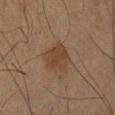The lesion was tiled from a total-body skin photograph and was not biopsied.
A 15 mm crop from a total-body photograph taken for skin-cancer surveillance.
The lesion is located on the left forearm.
Approximately 4 mm at its widest.
A male patient roughly 65 years of age.